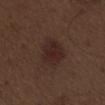  biopsy_status: not biopsied; imaged during a skin examination
  patient:
    sex: male
    age_approx: 70
  image:
    source: total-body photography crop
    field_of_view_mm: 15
  site: abdomen